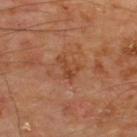| field | value |
|---|---|
| lighting | cross-polarized illumination |
| patient | male, in their mid- to late 60s |
| image source | ~15 mm crop, total-body skin-cancer survey |
| location | the upper back |
| size | ≈3 mm |
| image-analysis metrics | a footprint of about 4.5 mm², an eccentricity of roughly 0.75, and two-axis asymmetry of about 0.6; an average lesion color of about L≈43 a*≈24 b*≈34 (CIELAB), about 7 CIELAB-L* units darker than the surrounding skin, and a normalized border contrast of about 6; a nevus-likeness score of about 0/100 |